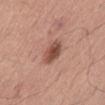No biopsy was performed on this lesion — it was imaged during a full skin examination and was not determined to be concerning.
On the abdomen.
A male subject aged 53 to 57.
Captured under white-light illumination.
This image is a 15 mm lesion crop taken from a total-body photograph.